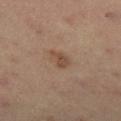Imaged during a routine full-body skin examination; the lesion was not biopsied and no histopathology is available. From the right lower leg. A male patient aged around 55. Imaged with cross-polarized lighting. A 15 mm close-up extracted from a 3D total-body photography capture.